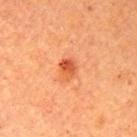  biopsy_status: not biopsied; imaged during a skin examination
  image:
    source: total-body photography crop
    field_of_view_mm: 15
  lesion_size:
    long_diameter_mm_approx: 2.5
  patient:
    sex: female
    age_approx: 60
  lighting: cross-polarized
  site: right upper arm